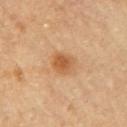Q: Illumination type?
A: cross-polarized illumination
Q: What kind of image is this?
A: ~15 mm crop, total-body skin-cancer survey
Q: What are the patient's age and sex?
A: female, roughly 65 years of age
Q: How large is the lesion?
A: about 2.5 mm
Q: Automated lesion metrics?
A: an area of roughly 6 mm², a shape eccentricity near 0.35, and a symmetry-axis asymmetry near 0.2; a lesion–skin lightness drop of about 10 and a normalized border contrast of about 7.5; a border-irregularity rating of about 1.5/10, a within-lesion color-variation index near 4/10, and peripheral color asymmetry of about 1.5; an automated nevus-likeness rating near 85 out of 100 and a detector confidence of about 100 out of 100 that the crop contains a lesion
Q: Lesion location?
A: the right upper arm